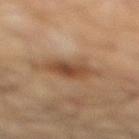This lesion was catalogued during total-body skin photography and was not selected for biopsy.
This is a cross-polarized tile.
The subject is a male aged approximately 65.
Automated tile analysis of the lesion measured border irregularity of about 4.5 on a 0–10 scale, internal color variation of about 4 on a 0–10 scale, and peripheral color asymmetry of about 1. The analysis additionally found a nevus-likeness score of about 60/100 and a lesion-detection confidence of about 100/100.
Located on the right lower leg.
A roughly 15 mm field-of-view crop from a total-body skin photograph.
Longest diameter approximately 6 mm.